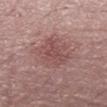This lesion was catalogued during total-body skin photography and was not selected for biopsy. Longest diameter approximately 5 mm. A male patient aged around 70. The total-body-photography lesion software estimated a mean CIELAB color near L≈49 a*≈22 b*≈21, about 8 CIELAB-L* units darker than the surrounding skin, and a normalized lesion–skin contrast near 5.5. And it measured a nevus-likeness score of about 30/100. A 15 mm close-up extracted from a 3D total-body photography capture. The lesion is on the abdomen.